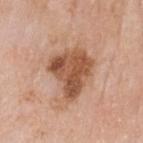{"biopsy_status": "not biopsied; imaged during a skin examination", "patient": {"sex": "male", "age_approx": 80}, "site": "head or neck", "image": {"source": "total-body photography crop", "field_of_view_mm": 15}}